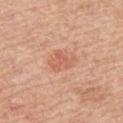Image and clinical context: A roughly 15 mm field-of-view crop from a total-body skin photograph. Automated tile analysis of the lesion measured an area of roughly 5.5 mm², a shape eccentricity near 0.7, and a shape-asymmetry score of about 0.45 (0 = symmetric). And it measured a lesion color around L≈61 a*≈26 b*≈32 in CIELAB and roughly 8 lightness units darker than nearby skin. A male subject aged 58 to 62. The lesion is on the upper back.